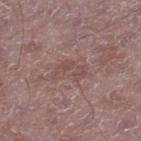| key | value |
|---|---|
| workup | catalogued during a skin exam; not biopsied |
| imaging modality | 15 mm crop, total-body photography |
| lesion diameter | about 3.5 mm |
| tile lighting | white-light illumination |
| site | the leg |
| image-analysis metrics | an automated nevus-likeness rating near 0 out of 100 and a detector confidence of about 70 out of 100 that the crop contains a lesion |
| patient | male, approximately 50 years of age |